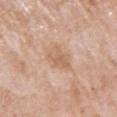Captured during whole-body skin photography for melanoma surveillance; the lesion was not biopsied. The recorded lesion diameter is about 3.5 mm. The lesion is located on the right upper arm. The subject is a female roughly 70 years of age. A roughly 15 mm field-of-view crop from a total-body skin photograph. The tile uses white-light illumination. An algorithmic analysis of the crop reported an area of roughly 7.5 mm², an eccentricity of roughly 0.5, and a shape-asymmetry score of about 0.25 (0 = symmetric). It also reported an average lesion color of about L≈63 a*≈19 b*≈32 (CIELAB).